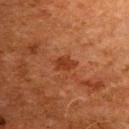<record>
<biopsy_status>not biopsied; imaged during a skin examination</biopsy_status>
<lighting>cross-polarized</lighting>
<site>chest</site>
<patient>
  <sex>male</sex>
  <age_approx>80</age_approx>
</patient>
<image>
  <source>total-body photography crop</source>
  <field_of_view_mm>15</field_of_view_mm>
</image>
</record>